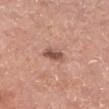Notes:
• workup: imaged on a skin check; not biopsied
• acquisition: ~15 mm crop, total-body skin-cancer survey
• body site: the leg
• lighting: white-light
• lesion diameter: about 3 mm
• subject: male, aged approximately 50